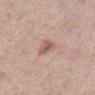Part of a total-body skin-imaging series; this lesion was reviewed on a skin check and was not flagged for biopsy. Located on the mid back. Imaged with white-light lighting. Automated image analysis of the tile measured a shape-asymmetry score of about 0.3 (0 = symmetric). And it measured roughly 10 lightness units darker than nearby skin and a normalized lesion–skin contrast near 7. It also reported border irregularity of about 3 on a 0–10 scale, a color-variation rating of about 2.5/10, and a peripheral color-asymmetry measure near 0.5. The recorded lesion diameter is about 3.5 mm. A male subject, in their 70s. A 15 mm crop from a total-body photograph taken for skin-cancer surveillance.